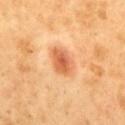Impression:
Part of a total-body skin-imaging series; this lesion was reviewed on a skin check and was not flagged for biopsy.
Clinical summary:
The recorded lesion diameter is about 3.5 mm. A 15 mm crop from a total-body photograph taken for skin-cancer surveillance. From the mid back. The patient is a male aged approximately 50. Imaged with cross-polarized lighting.